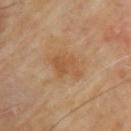biopsy status = total-body-photography surveillance lesion; no biopsy | subject = male, roughly 65 years of age | tile lighting = cross-polarized illumination | imaging modality = total-body-photography crop, ~15 mm field of view | lesion size = about 4 mm | body site = the right upper arm | automated lesion analysis = a lesion color around L≈54 a*≈20 b*≈37 in CIELAB, a lesion–skin lightness drop of about 7, and a normalized lesion–skin contrast near 6; a border-irregularity rating of about 3/10, a color-variation rating of about 3/10, and peripheral color asymmetry of about 1.